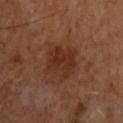Impression:
Captured during whole-body skin photography for melanoma surveillance; the lesion was not biopsied.
Acquisition and patient details:
The patient is a male aged 63 to 67. A lesion tile, about 15 mm wide, cut from a 3D total-body photograph.